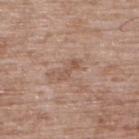Q: Was a biopsy performed?
A: imaged on a skin check; not biopsied
Q: Lesion location?
A: the upper back
Q: Illumination type?
A: white-light
Q: What are the patient's age and sex?
A: male, aged 48 to 52
Q: What kind of image is this?
A: ~15 mm tile from a whole-body skin photo